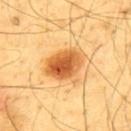follow-up — catalogued during a skin exam; not biopsied
image source — 15 mm crop, total-body photography
patient — male, aged approximately 65
diameter — about 4.5 mm
illumination — cross-polarized illumination
anatomic site — the upper back